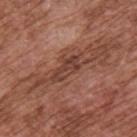Findings:
• workup: total-body-photography surveillance lesion; no biopsy
• acquisition: ~15 mm crop, total-body skin-cancer survey
• site: the upper back
• patient: male, in their mid-70s